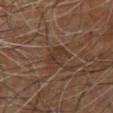workup: imaged on a skin check; not biopsied.